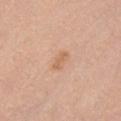workup — no biopsy performed (imaged during a skin exam)
imaging modality — 15 mm crop, total-body photography
subject — female, approximately 40 years of age
illumination — white-light illumination
size — ≈3 mm
location — the front of the torso